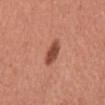<tbp_lesion>
<lesion_size>
  <long_diameter_mm_approx>3.5</long_diameter_mm_approx>
</lesion_size>
<automated_metrics>
  <cielab_L>48</cielab_L>
  <cielab_a>28</cielab_a>
  <cielab_b>30</cielab_b>
  <vs_skin_contrast_norm>9.5</vs_skin_contrast_norm>
  <border_irregularity_0_10>1.5</border_irregularity_0_10>
  <color_variation_0_10>2.5</color_variation_0_10>
  <peripheral_color_asymmetry>1.0</peripheral_color_asymmetry>
  <nevus_likeness_0_100>90</nevus_likeness_0_100>
  <lesion_detection_confidence_0_100>100</lesion_detection_confidence_0_100>
</automated_metrics>
<patient>
  <sex>male</sex>
  <age_approx>45</age_approx>
</patient>
<site>abdomen</site>
<image>
  <source>total-body photography crop</source>
  <field_of_view_mm>15</field_of_view_mm>
</image>
<lighting>white-light</lighting>
</tbp_lesion>